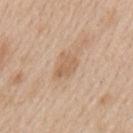biopsy status: catalogued during a skin exam; not biopsied
anatomic site: the mid back
automated lesion analysis: an outline eccentricity of about 0.75 (0 = round, 1 = elongated) and a symmetry-axis asymmetry near 0.3; a mean CIELAB color near L≈61 a*≈18 b*≈33, a lesion–skin lightness drop of about 8, and a normalized lesion–skin contrast near 6; a border-irregularity rating of about 3/10, a color-variation rating of about 2/10, and peripheral color asymmetry of about 1; a nevus-likeness score of about 10/100 and a detector confidence of about 100 out of 100 that the crop contains a lesion
patient: male, about 60 years old
imaging modality: 15 mm crop, total-body photography